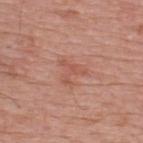image:
  source: total-body photography crop
  field_of_view_mm: 15
site: upper back
lesion_size:
  long_diameter_mm_approx: 3.0
patient:
  sex: male
  age_approx: 75
automated_metrics:
  area_mm2_approx: 4.0
  eccentricity: 0.3
  shape_asymmetry: 0.8
  cielab_L: 54
  cielab_a: 26
  cielab_b: 29
  vs_skin_darker_L: 7.0
  vs_skin_contrast_norm: 5.0
  nevus_likeness_0_100: 5
  lesion_detection_confidence_0_100: 100